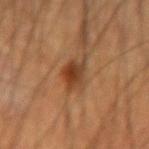{"biopsy_status": "not biopsied; imaged during a skin examination", "lighting": "cross-polarized", "lesion_size": {"long_diameter_mm_approx": 3.5}, "image": {"source": "total-body photography crop", "field_of_view_mm": 15}, "patient": {"sex": "male", "age_approx": 55}, "site": "right forearm"}